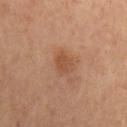The lesion was photographed on a routine skin check and not biopsied; there is no pathology result.
The recorded lesion diameter is about 3 mm.
A male subject, aged around 60.
Captured under cross-polarized illumination.
The lesion is on the mid back.
Automated tile analysis of the lesion measured a lesion area of about 5 mm², an outline eccentricity of about 0.7 (0 = round, 1 = elongated), and two-axis asymmetry of about 0.2. The software also gave roughly 7 lightness units darker than nearby skin and a lesion-to-skin contrast of about 6 (normalized; higher = more distinct). The software also gave border irregularity of about 2 on a 0–10 scale. And it measured a classifier nevus-likeness of about 15/100 and a lesion-detection confidence of about 100/100.
Cropped from a total-body skin-imaging series; the visible field is about 15 mm.